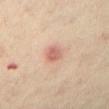image source = total-body-photography crop, ~15 mm field of view | TBP lesion metrics = a nevus-likeness score of about 20/100 | lesion diameter = ~3 mm (longest diameter) | patient = female, aged around 55 | site = the leg.